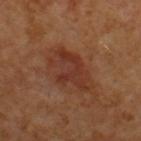patient: male, aged 58–62 | size: about 6 mm | automated metrics: an area of roughly 14 mm² and a shape eccentricity near 0.8; a within-lesion color-variation index near 4/10 and radial color variation of about 1.5; a nevus-likeness score of about 5/100 and lesion-presence confidence of about 100/100 | body site: the upper back | acquisition: total-body-photography crop, ~15 mm field of view | histopathologic diagnosis: a melanoma in situ arising in association with a nevus.